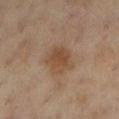biopsy_status: not biopsied; imaged during a skin examination
lighting: cross-polarized
image:
  source: total-body photography crop
  field_of_view_mm: 15
site: left lower leg
lesion_size:
  long_diameter_mm_approx: 3.5
patient:
  sex: female
  age_approx: 55